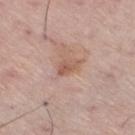Clinical impression: The lesion was photographed on a routine skin check and not biopsied; there is no pathology result. Background: About 2.5 mm across. The tile uses white-light illumination. This image is a 15 mm lesion crop taken from a total-body photograph. The patient is a male aged approximately 70. On the right thigh. An algorithmic analysis of the crop reported a footprint of about 3.5 mm², an eccentricity of roughly 0.75, and two-axis asymmetry of about 0.4. And it measured a within-lesion color-variation index near 2/10 and radial color variation of about 0.5. The analysis additionally found a lesion-detection confidence of about 100/100.